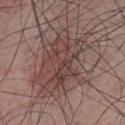Recorded during total-body skin imaging; not selected for excision or biopsy.
On the chest.
A close-up tile cropped from a whole-body skin photograph, about 15 mm across.
The tile uses white-light illumination.
The patient is a male aged around 65.
About 7 mm across.